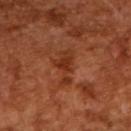No biopsy was performed on this lesion — it was imaged during a full skin examination and was not determined to be concerning. A 15 mm close-up extracted from a 3D total-body photography capture. A male subject aged 63–67.